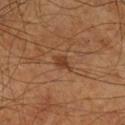The lesion was photographed on a routine skin check and not biopsied; there is no pathology result. Cropped from a total-body skin-imaging series; the visible field is about 15 mm. The lesion is located on the left leg. The tile uses cross-polarized illumination. A male patient, about 65 years old.